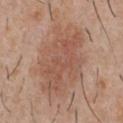follow-up: total-body-photography surveillance lesion; no biopsy | anatomic site: the chest | size: ~9 mm (longest diameter) | subject: male, aged 28 to 32 | acquisition: total-body-photography crop, ~15 mm field of view | automated metrics: a lesion area of about 38 mm², an outline eccentricity of about 0.8 (0 = round, 1 = elongated), and a shape-asymmetry score of about 0.15 (0 = symmetric); a mean CIELAB color near L≈54 a*≈20 b*≈29, a lesion–skin lightness drop of about 8, and a normalized border contrast of about 6; a border-irregularity index near 3/10, a within-lesion color-variation index near 4/10, and peripheral color asymmetry of about 1.5 | tile lighting: white-light illumination.